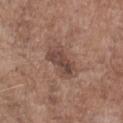<lesion>
<biopsy_status>not biopsied; imaged during a skin examination</biopsy_status>
<site>chest</site>
<lighting>white-light</lighting>
<image>
  <source>total-body photography crop</source>
  <field_of_view_mm>15</field_of_view_mm>
</image>
<automated_metrics>
  <cielab_L>45</cielab_L>
  <cielab_a>18</cielab_a>
  <cielab_b>23</cielab_b>
  <vs_skin_contrast_norm>7.5</vs_skin_contrast_norm>
  <color_variation_0_10>4.5</color_variation_0_10>
  <nevus_likeness_0_100>10</nevus_likeness_0_100>
  <lesion_detection_confidence_0_100>100</lesion_detection_confidence_0_100>
</automated_metrics>
<patient>
  <sex>male</sex>
  <age_approx>70</age_approx>
</patient>
<lesion_size>
  <long_diameter_mm_approx>4.5</long_diameter_mm_approx>
</lesion_size>
</lesion>